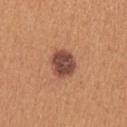| feature | finding |
|---|---|
| notes | total-body-photography surveillance lesion; no biopsy |
| acquisition | 15 mm crop, total-body photography |
| lighting | white-light |
| anatomic site | the left upper arm |
| patient | female, approximately 40 years of age |
| size | ≈3.5 mm |